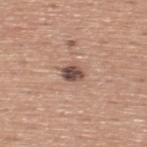Impression: This lesion was catalogued during total-body skin photography and was not selected for biopsy. Context: A male patient, aged approximately 45. The tile uses white-light illumination. On the back. Measured at roughly 2.5 mm in maximum diameter. This image is a 15 mm lesion crop taken from a total-body photograph.